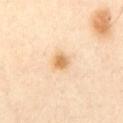biopsy status: no biopsy performed (imaged during a skin exam) | lighting: cross-polarized | anatomic site: the chest | acquisition: total-body-photography crop, ~15 mm field of view | subject: male, aged 53 to 57.